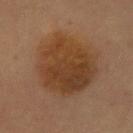Findings:
* follow-up: no biopsy performed (imaged during a skin exam)
* size: about 9 mm
* anatomic site: the abdomen
* image: 15 mm crop, total-body photography
* tile lighting: cross-polarized illumination
* TBP lesion metrics: a mean CIELAB color near L≈34 a*≈17 b*≈29, about 8 CIELAB-L* units darker than the surrounding skin, and a normalized lesion–skin contrast near 8.5; border irregularity of about 2 on a 0–10 scale and peripheral color asymmetry of about 1.5
* subject: female, aged 58–62